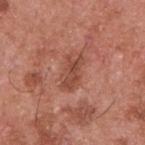Notes:
• size — about 4 mm
• lighting — white-light illumination
• image — ~15 mm tile from a whole-body skin photo
• automated lesion analysis — an area of roughly 8 mm² and an outline eccentricity of about 0.85 (0 = round, 1 = elongated)
• subject — male, aged approximately 55
• location — the upper back